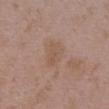patient: female, roughly 35 years of age; image: ~15 mm tile from a whole-body skin photo; anatomic site: the chest.